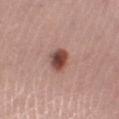From the left lower leg.
The patient is a female aged 53–57.
A lesion tile, about 15 mm wide, cut from a 3D total-body photograph.